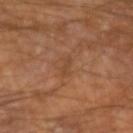Impression:
Part of a total-body skin-imaging series; this lesion was reviewed on a skin check and was not flagged for biopsy.
Background:
Longest diameter approximately 2.5 mm. From the left forearm. The subject is a male roughly 65 years of age. This image is a 15 mm lesion crop taken from a total-body photograph. The tile uses cross-polarized illumination. An algorithmic analysis of the crop reported a footprint of about 1.5 mm² and a shape-asymmetry score of about 0.35 (0 = symmetric). The software also gave a mean CIELAB color near L≈41 a*≈19 b*≈31, a lesion–skin lightness drop of about 6, and a normalized lesion–skin contrast near 5. The software also gave border irregularity of about 5 on a 0–10 scale, a within-lesion color-variation index near 0/10, and a peripheral color-asymmetry measure near 0. And it measured an automated nevus-likeness rating near 0 out of 100 and a detector confidence of about 95 out of 100 that the crop contains a lesion.